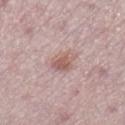Q: Was a biopsy performed?
A: imaged on a skin check; not biopsied
Q: Who is the patient?
A: female, aged approximately 50
Q: Lesion size?
A: about 3.5 mm
Q: How was this image acquired?
A: ~15 mm tile from a whole-body skin photo
Q: Lesion location?
A: the right lower leg
Q: What did automated image analysis measure?
A: an average lesion color of about L≈58 a*≈20 b*≈22 (CIELAB) and a normalized lesion–skin contrast near 7; internal color variation of about 3 on a 0–10 scale; a nevus-likeness score of about 30/100